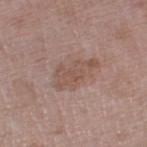Imaged during a routine full-body skin examination; the lesion was not biopsied and no histopathology is available.
From the leg.
This is a white-light tile.
Cropped from a whole-body photographic skin survey; the tile spans about 15 mm.
The recorded lesion diameter is about 5 mm.
A male patient aged 68 to 72.